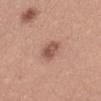Impression: Captured during whole-body skin photography for melanoma surveillance; the lesion was not biopsied. Acquisition and patient details: The lesion's longest dimension is about 3 mm. This is a white-light tile. The patient is a female aged around 35. On the front of the torso. A 15 mm close-up extracted from a 3D total-body photography capture.